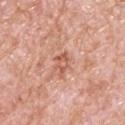Notes:
• automated lesion analysis · a border-irregularity index near 4.5/10 and peripheral color asymmetry of about 1; a classifier nevus-likeness of about 0/100 and lesion-presence confidence of about 100/100
• acquisition · total-body-photography crop, ~15 mm field of view
• diameter · ≈2.5 mm
• subject · male, aged 78–82
• location · the back